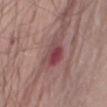workup: total-body-photography surveillance lesion; no biopsy
body site: the chest
patient: male, in their mid-80s or older
image source: ~15 mm tile from a whole-body skin photo
lesion diameter: about 5 mm
illumination: white-light